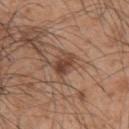Image and clinical context: Cropped from a total-body skin-imaging series; the visible field is about 15 mm. On the upper back. The subject is a male aged around 45. Approximately 3 mm at its widest.